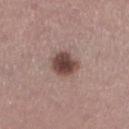This image is a 15 mm lesion crop taken from a total-body photograph. On the left lower leg. An algorithmic analysis of the crop reported an average lesion color of about L≈44 a*≈19 b*≈21 (CIELAB) and about 16 CIELAB-L* units darker than the surrounding skin. And it measured a border-irregularity index near 1.5/10, a within-lesion color-variation index near 4/10, and peripheral color asymmetry of about 1. And it measured an automated nevus-likeness rating near 95 out of 100 and a detector confidence of about 100 out of 100 that the crop contains a lesion. The recorded lesion diameter is about 3.5 mm. A male patient aged approximately 45.